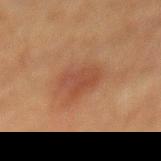Imaged during a routine full-body skin examination; the lesion was not biopsied and no histopathology is available.
A close-up tile cropped from a whole-body skin photograph, about 15 mm across.
The patient is a male in their 50s.
The lesion's longest dimension is about 3.5 mm.
The lesion is on the mid back.
This is a cross-polarized tile.